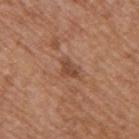| feature | finding |
|---|---|
| follow-up | imaged on a skin check; not biopsied |
| tile lighting | white-light illumination |
| lesion size | ≈3 mm |
| anatomic site | the right upper arm |
| automated lesion analysis | a lesion color around L≈47 a*≈22 b*≈30 in CIELAB, roughly 9 lightness units darker than nearby skin, and a normalized border contrast of about 7; a border-irregularity index near 4.5/10, a color-variation rating of about 1.5/10, and radial color variation of about 0.5; an automated nevus-likeness rating near 0 out of 100 and a lesion-detection confidence of about 100/100 |
| subject | male, roughly 65 years of age |
| image source | ~15 mm crop, total-body skin-cancer survey |